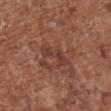Q: Was this lesion biopsied?
A: total-body-photography surveillance lesion; no biopsy
Q: What lighting was used for the tile?
A: white-light
Q: Where on the body is the lesion?
A: the upper back
Q: What are the patient's age and sex?
A: female, aged 73 to 77
Q: What is the lesion's diameter?
A: ≈4 mm
Q: What kind of image is this?
A: ~15 mm tile from a whole-body skin photo